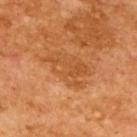<tbp_lesion>
  <biopsy_status>not biopsied; imaged during a skin examination</biopsy_status>
  <lesion_size>
    <long_diameter_mm_approx>6.0</long_diameter_mm_approx>
  </lesion_size>
  <patient>
    <sex>male</sex>
    <age_approx>65</age_approx>
  </patient>
  <lighting>cross-polarized</lighting>
  <image>
    <source>total-body photography crop</source>
    <field_of_view_mm>15</field_of_view_mm>
  </image>
</tbp_lesion>